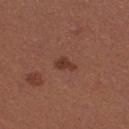<tbp_lesion>
<biopsy_status>not biopsied; imaged during a skin examination</biopsy_status>
<site>right thigh</site>
<patient>
  <sex>female</sex>
  <age_approx>25</age_approx>
</patient>
<image>
  <source>total-body photography crop</source>
  <field_of_view_mm>15</field_of_view_mm>
</image>
<lesion_size>
  <long_diameter_mm_approx>2.5</long_diameter_mm_approx>
</lesion_size>
<lighting>white-light</lighting>
</tbp_lesion>